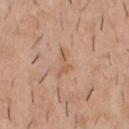follow-up: imaged on a skin check; not biopsied | patient: male, about 40 years old | site: the chest | image source: ~15 mm tile from a whole-body skin photo.